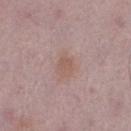Findings:
– follow-up — catalogued during a skin exam; not biopsied
– automated lesion analysis — an area of roughly 4.5 mm², an eccentricity of roughly 0.55, and a shape-asymmetry score of about 0.25 (0 = symmetric); a mean CIELAB color near L≈56 a*≈19 b*≈23, a lesion–skin lightness drop of about 6, and a normalized lesion–skin contrast near 5.5; radial color variation of about 0.5
– image — ~15 mm crop, total-body skin-cancer survey
– subject — male, aged 48 to 52
– site — the leg
– diameter — about 3 mm
– illumination — white-light illumination